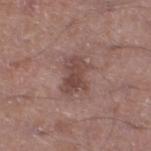The lesion was tiled from a total-body skin photograph and was not biopsied.
Located on the left lower leg.
The patient is a male aged 53–57.
A 15 mm close-up tile from a total-body photography series done for melanoma screening.